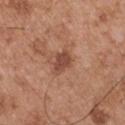patient = male, in their mid-50s
anatomic site = the left upper arm
tile lighting = white-light
imaging modality = ~15 mm crop, total-body skin-cancer survey